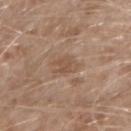Q: Was this lesion biopsied?
A: no biopsy performed (imaged during a skin exam)
Q: Who is the patient?
A: male, aged 58 to 62
Q: Illumination type?
A: white-light
Q: How was this image acquired?
A: ~15 mm tile from a whole-body skin photo
Q: What did automated image analysis measure?
A: a lesion color around L≈51 a*≈17 b*≈29 in CIELAB, a lesion–skin lightness drop of about 7, and a normalized lesion–skin contrast near 5.5; an automated nevus-likeness rating near 0 out of 100 and a lesion-detection confidence of about 100/100
Q: What is the lesion's diameter?
A: about 3 mm
Q: What is the anatomic site?
A: the right forearm